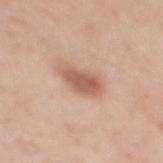biopsy status — total-body-photography surveillance lesion; no biopsy | image source — ~15 mm crop, total-body skin-cancer survey | patient — female, approximately 35 years of age | illumination — white-light | location — the back | lesion diameter — ≈4.5 mm.